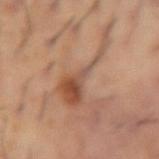Imaged during a routine full-body skin examination; the lesion was not biopsied and no histopathology is available. A male patient, aged 53 to 57. About 8.5 mm across. On the left forearm. The tile uses cross-polarized illumination. A 15 mm crop from a total-body photograph taken for skin-cancer surveillance.